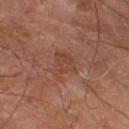Case summary:
– tile lighting: cross-polarized illumination
– image: ~15 mm tile from a whole-body skin photo
– patient: male, aged around 70
– diameter: ~4 mm (longest diameter)
– site: the left lower leg